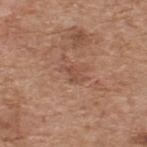Clinical summary: The lesion is on the back. This is a white-light tile. A close-up tile cropped from a whole-body skin photograph, about 15 mm across. Measured at roughly 3 mm in maximum diameter. The lesion-visualizer software estimated an average lesion color of about L≈49 a*≈22 b*≈29 (CIELAB), a lesion–skin lightness drop of about 7, and a normalized border contrast of about 5. It also reported an automated nevus-likeness rating near 0 out of 100 and lesion-presence confidence of about 100/100. A male patient, aged 58–62.